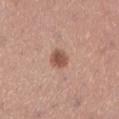follow-up: catalogued during a skin exam; not biopsied
imaging modality: total-body-photography crop, ~15 mm field of view
subject: female, aged approximately 30
diameter: ≈2.5 mm
illumination: white-light illumination
body site: the left lower leg